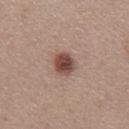Notes:
- biopsy status: total-body-photography surveillance lesion; no biopsy
- patient: male, aged around 55
- diameter: about 3 mm
- image-analysis metrics: an eccentricity of roughly 0.65 and a symmetry-axis asymmetry near 0.2; a mean CIELAB color near L≈46 a*≈21 b*≈23; a border-irregularity rating of about 1.5/10 and internal color variation of about 5.5 on a 0–10 scale; lesion-presence confidence of about 100/100
- image: ~15 mm tile from a whole-body skin photo
- tile lighting: white-light illumination
- location: the abdomen